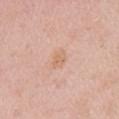Imaged during a routine full-body skin examination; the lesion was not biopsied and no histopathology is available. Longest diameter approximately 2.5 mm. The tile uses white-light illumination. A female patient, aged 48–52. A close-up tile cropped from a whole-body skin photograph, about 15 mm across. From the right upper arm. Automated image analysis of the tile measured an area of roughly 3.5 mm², an outline eccentricity of about 0.75 (0 = round, 1 = elongated), and a shape-asymmetry score of about 0.35 (0 = symmetric). The software also gave a mean CIELAB color near L≈66 a*≈21 b*≈32, about 6 CIELAB-L* units darker than the surrounding skin, and a lesion-to-skin contrast of about 5.5 (normalized; higher = more distinct).